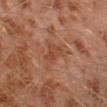Case summary:
- biopsy status · no biopsy performed (imaged during a skin exam)
- subject · male, in their 30s
- image source · 15 mm crop, total-body photography
- lighting · cross-polarized illumination
- location · the left lower leg